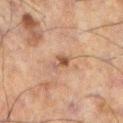Q: Was a biopsy performed?
A: catalogued during a skin exam; not biopsied
Q: What did automated image analysis measure?
A: a lesion area of about 3.5 mm², an outline eccentricity of about 0.85 (0 = round, 1 = elongated), and a shape-asymmetry score of about 0.35 (0 = symmetric)
Q: Where on the body is the lesion?
A: the left lower leg
Q: Who is the patient?
A: male, about 60 years old
Q: What is the imaging modality?
A: ~15 mm tile from a whole-body skin photo